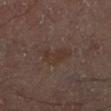- biopsy status · total-body-photography surveillance lesion; no biopsy
- TBP lesion metrics · an average lesion color of about L≈28 a*≈14 b*≈19 (CIELAB), about 4 CIELAB-L* units darker than the surrounding skin, and a normalized border contrast of about 5.5; a border-irregularity rating of about 5/10, internal color variation of about 2 on a 0–10 scale, and a peripheral color-asymmetry measure near 0.5; an automated nevus-likeness rating near 0 out of 100 and a detector confidence of about 100 out of 100 that the crop contains a lesion
- image source · ~15 mm tile from a whole-body skin photo
- patient · male, aged 48–52
- anatomic site · the right lower leg
- size · about 5 mm
- lighting · cross-polarized